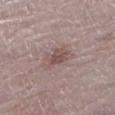<case>
  <biopsy_status>not biopsied; imaged during a skin examination</biopsy_status>
  <image>
    <source>total-body photography crop</source>
    <field_of_view_mm>15</field_of_view_mm>
  </image>
  <patient>
    <sex>female</sex>
    <age_approx>65</age_approx>
  </patient>
  <site>leg</site>
</case>